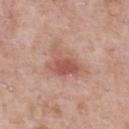<tbp_lesion>
  <biopsy_status>not biopsied; imaged during a skin examination</biopsy_status>
  <lesion_size>
    <long_diameter_mm_approx>5.0</long_diameter_mm_approx>
  </lesion_size>
  <site>chest</site>
  <lighting>white-light</lighting>
  <image>
    <source>total-body photography crop</source>
    <field_of_view_mm>15</field_of_view_mm>
  </image>
  <patient>
    <sex>male</sex>
    <age_approx>55</age_approx>
  </patient>
</tbp_lesion>